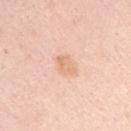Case summary:
* workup: total-body-photography surveillance lesion; no biopsy
* imaging modality: total-body-photography crop, ~15 mm field of view
* body site: the left upper arm
* image-analysis metrics: an outline eccentricity of about 0.75 (0 = round, 1 = elongated) and a symmetry-axis asymmetry near 0.25; a lesion color around L≈74 a*≈20 b*≈35 in CIELAB, about 7 CIELAB-L* units darker than the surrounding skin, and a normalized lesion–skin contrast near 5.5; internal color variation of about 2 on a 0–10 scale and a peripheral color-asymmetry measure near 0.5; a classifier nevus-likeness of about 10/100 and lesion-presence confidence of about 100/100
* subject: female, in their 40s
* tile lighting: white-light
* diameter: ≈3 mm